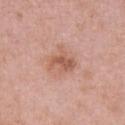A female patient, approximately 30 years of age.
Approximately 3.5 mm at its widest.
Automated tile analysis of the lesion measured an average lesion color of about L≈57 a*≈24 b*≈29 (CIELAB), about 9 CIELAB-L* units darker than the surrounding skin, and a lesion-to-skin contrast of about 6.5 (normalized; higher = more distinct).
The lesion is located on the upper back.
Cropped from a whole-body photographic skin survey; the tile spans about 15 mm.